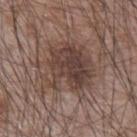Case summary:
- workup: imaged on a skin check; not biopsied
- diameter: ~7 mm (longest diameter)
- image source: total-body-photography crop, ~15 mm field of view
- location: the left forearm
- subject: male, approximately 75 years of age
- illumination: white-light illumination